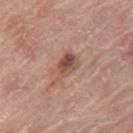Clinical impression: No biopsy was performed on this lesion — it was imaged during a full skin examination and was not determined to be concerning. Background: Measured at roughly 4.5 mm in maximum diameter. The lesion is located on the leg. A female patient aged 58–62. A lesion tile, about 15 mm wide, cut from a 3D total-body photograph.